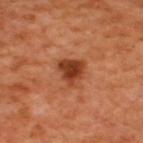Clinical impression:
Captured during whole-body skin photography for melanoma surveillance; the lesion was not biopsied.
Context:
Imaged with cross-polarized lighting. Approximately 3.5 mm at its widest. The patient is a male approximately 50 years of age. A 15 mm crop from a total-body photograph taken for skin-cancer surveillance. The lesion is located on the upper back.